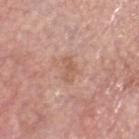notes: catalogued during a skin exam; not biopsied
tile lighting: white-light illumination
diameter: ≈3 mm
patient: male, about 60 years old
image: total-body-photography crop, ~15 mm field of view
automated metrics: a footprint of about 3.5 mm² and a symmetry-axis asymmetry near 0.3; a mean CIELAB color near L≈58 a*≈21 b*≈29, about 8 CIELAB-L* units darker than the surrounding skin, and a lesion-to-skin contrast of about 5.5 (normalized; higher = more distinct)
anatomic site: the head or neck